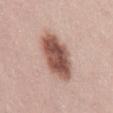The lesion is located on the mid back.
A male patient, roughly 45 years of age.
A roughly 15 mm field-of-view crop from a total-body skin photograph.